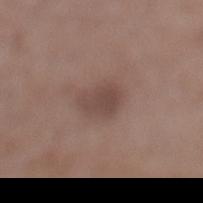  biopsy_status: not biopsied; imaged during a skin examination
  image:
    source: total-body photography crop
    field_of_view_mm: 15
  lighting: white-light
  patient:
    sex: male
    age_approx: 50
  lesion_size:
    long_diameter_mm_approx: 3.5
  automated_metrics:
    shape_asymmetry: 0.25
    vs_skin_darker_L: 8.0
    vs_skin_contrast_norm: 6.5
  site: left lower leg